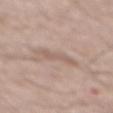notes: catalogued during a skin exam; not biopsied | illumination: white-light | diameter: ≈4 mm | TBP lesion metrics: a shape-asymmetry score of about 0.4 (0 = symmetric); a lesion color around L≈59 a*≈16 b*≈25 in CIELAB and about 7 CIELAB-L* units darker than the surrounding skin | patient: male, aged approximately 55 | image: total-body-photography crop, ~15 mm field of view | body site: the back.